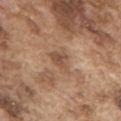Clinical impression:
Imaged during a routine full-body skin examination; the lesion was not biopsied and no histopathology is available.
Context:
The subject is a male aged around 75. Located on the upper back. Captured under white-light illumination. Cropped from a total-body skin-imaging series; the visible field is about 15 mm. The lesion's longest dimension is about 3.5 mm.